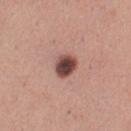biopsy_status: not biopsied; imaged during a skin examination
lesion_size:
  long_diameter_mm_approx: 2.5
patient:
  sex: female
  age_approx: 30
lighting: white-light
automated_metrics:
  cielab_L: 45
  cielab_a: 22
  cielab_b: 23
  vs_skin_darker_L: 19.0
  vs_skin_contrast_norm: 13.0
  lesion_detection_confidence_0_100: 100
image:
  source: total-body photography crop
  field_of_view_mm: 15
site: left thigh